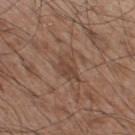Assessment: No biopsy was performed on this lesion — it was imaged during a full skin examination and was not determined to be concerning. Context: A close-up tile cropped from a whole-body skin photograph, about 15 mm across. The subject is a male in their mid-50s. The lesion is on the leg. Captured under white-light illumination. The lesion-visualizer software estimated an area of roughly 5.5 mm², an outline eccentricity of about 0.75 (0 = round, 1 = elongated), and two-axis asymmetry of about 0.3. The software also gave a mean CIELAB color near L≈43 a*≈18 b*≈27, roughly 7 lightness units darker than nearby skin, and a normalized lesion–skin contrast near 6. Measured at roughly 3.5 mm in maximum diameter.